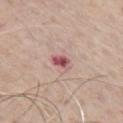Case summary:
– follow-up · no biopsy performed (imaged during a skin exam)
– diameter · about 2.5 mm
– lighting · white-light illumination
– anatomic site · the chest
– imaging modality · 15 mm crop, total-body photography
– patient · male, approximately 60 years of age